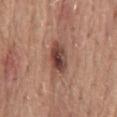Part of a total-body skin-imaging series; this lesion was reviewed on a skin check and was not flagged for biopsy. The lesion is on the lower back. A roughly 15 mm field-of-view crop from a total-body skin photograph. A male subject, aged around 55.